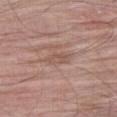notes: catalogued during a skin exam; not biopsied
subject: male, in their 80s
illumination: white-light illumination
site: the right thigh
size: about 3 mm
automated metrics: a lesion area of about 4.5 mm² and two-axis asymmetry of about 0.2; a border-irregularity rating of about 2.5/10, a color-variation rating of about 2.5/10, and peripheral color asymmetry of about 1
image source: total-body-photography crop, ~15 mm field of view